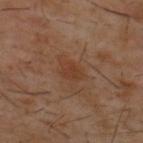Recorded during total-body skin imaging; not selected for excision or biopsy. The lesion is on the upper back. Cropped from a whole-body photographic skin survey; the tile spans about 15 mm. The lesion-visualizer software estimated a classifier nevus-likeness of about 35/100 and a detector confidence of about 100 out of 100 that the crop contains a lesion. A male patient, about 60 years old. Approximately 3 mm at its widest.